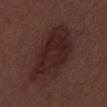The patient is a male aged 28 to 32.
On the mid back.
A 15 mm crop from a total-body photograph taken for skin-cancer surveillance.
Longest diameter approximately 9 mm.
This is a white-light tile.